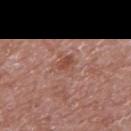Approximately 3 mm at its widest.
This is a white-light tile.
Automated image analysis of the tile measured a footprint of about 6.5 mm², an outline eccentricity of about 0.3 (0 = round, 1 = elongated), and a symmetry-axis asymmetry near 0.3. The analysis additionally found a detector confidence of about 100 out of 100 that the crop contains a lesion.
A 15 mm close-up extracted from a 3D total-body photography capture.
A male subject, in their 70s.
The lesion is located on the upper back.